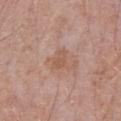Impression:
The lesion was photographed on a routine skin check and not biopsied; there is no pathology result.
Context:
The lesion is located on the chest. The recorded lesion diameter is about 2.5 mm. Automated tile analysis of the lesion measured an area of roughly 4.5 mm², an eccentricity of roughly 0.75, and a shape-asymmetry score of about 0.35 (0 = symmetric). It also reported border irregularity of about 3.5 on a 0–10 scale, a color-variation rating of about 2/10, and radial color variation of about 0.5. A roughly 15 mm field-of-view crop from a total-body skin photograph. The patient is a male approximately 70 years of age.